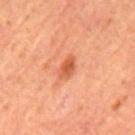Imaged during a routine full-body skin examination; the lesion was not biopsied and no histopathology is available.
On the mid back.
A male patient in their mid-60s.
A roughly 15 mm field-of-view crop from a total-body skin photograph.